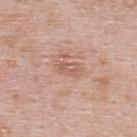A 15 mm close-up tile from a total-body photography series done for melanoma screening. On the upper back. A male patient, in their 40s. The tile uses white-light illumination. Approximately 2.5 mm at its widest.